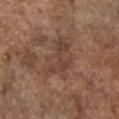{"biopsy_status": "not biopsied; imaged during a skin examination", "automated_metrics": {"area_mm2_approx": 14.0, "eccentricity": 0.8, "vs_skin_darker_L": 7.0, "vs_skin_contrast_norm": 5.5}, "lighting": "white-light", "image": {"source": "total-body photography crop", "field_of_view_mm": 15}, "lesion_size": {"long_diameter_mm_approx": 5.5}, "site": "chest", "patient": {"sex": "male", "age_approx": 75}}